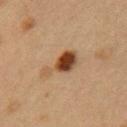Impression:
This lesion was catalogued during total-body skin photography and was not selected for biopsy.
Context:
The patient is a female approximately 40 years of age. The lesion's longest dimension is about 4.5 mm. Automated tile analysis of the lesion measured a footprint of about 8 mm², an eccentricity of roughly 0.8, and two-axis asymmetry of about 0.5. It also reported about 13 CIELAB-L* units darker than the surrounding skin and a normalized lesion–skin contrast near 11. It also reported a border-irregularity index near 5.5/10, internal color variation of about 6.5 on a 0–10 scale, and peripheral color asymmetry of about 2. A roughly 15 mm field-of-view crop from a total-body skin photograph. Located on the left upper arm. Captured under cross-polarized illumination.